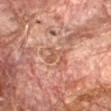{"biopsy_status": "not biopsied; imaged during a skin examination", "image": {"source": "total-body photography crop", "field_of_view_mm": 15}, "patient": {"sex": "male", "age_approx": 80}, "automated_metrics": {"area_mm2_approx": 4.0, "eccentricity": 0.7, "border_irregularity_0_10": 5.5, "color_variation_0_10": 0.0, "peripheral_color_asymmetry": 0.0, "nevus_likeness_0_100": 0, "lesion_detection_confidence_0_100": 60}, "lighting": "cross-polarized", "site": "right forearm"}